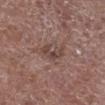Assessment:
Part of a total-body skin-imaging series; this lesion was reviewed on a skin check and was not flagged for biopsy.
Clinical summary:
The lesion's longest dimension is about 3.5 mm. The lesion-visualizer software estimated a lesion area of about 4.5 mm², an outline eccentricity of about 0.85 (0 = round, 1 = elongated), and two-axis asymmetry of about 0.45. The analysis additionally found an average lesion color of about L≈43 a*≈18 b*≈20 (CIELAB), about 8 CIELAB-L* units darker than the surrounding skin, and a normalized lesion–skin contrast near 7. Captured under white-light illumination. A male subject, roughly 70 years of age. A lesion tile, about 15 mm wide, cut from a 3D total-body photograph. The lesion is located on the right lower leg.